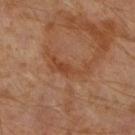{
  "biopsy_status": "not biopsied; imaged during a skin examination",
  "lesion_size": {
    "long_diameter_mm_approx": 5.0
  },
  "image": {
    "source": "total-body photography crop",
    "field_of_view_mm": 15
  },
  "automated_metrics": {
    "cielab_L": 41,
    "cielab_a": 22,
    "cielab_b": 31,
    "vs_skin_darker_L": 6.0,
    "vs_skin_contrast_norm": 6.0,
    "color_variation_0_10": 2.0,
    "peripheral_color_asymmetry": 0.5,
    "nevus_likeness_0_100": 0,
    "lesion_detection_confidence_0_100": 100
  },
  "patient": {
    "sex": "male",
    "age_approx": 60
  },
  "lighting": "cross-polarized",
  "site": "right lower leg"
}